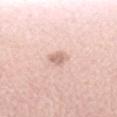{
  "image": {
    "source": "total-body photography crop",
    "field_of_view_mm": 15
  },
  "site": "left forearm",
  "patient": {
    "sex": "female",
    "age_approx": 40
  },
  "lesion_size": {
    "long_diameter_mm_approx": 2.5
  },
  "lighting": "white-light"
}